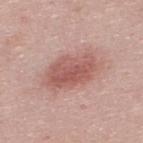{
  "biopsy_status": "not biopsied; imaged during a skin examination",
  "image": {
    "source": "total-body photography crop",
    "field_of_view_mm": 15
  },
  "lighting": "white-light",
  "site": "upper back",
  "patient": {
    "sex": "male",
    "age_approx": 25
  },
  "lesion_size": {
    "long_diameter_mm_approx": 6.0
  }
}